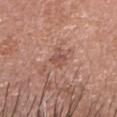The lesion was tiled from a total-body skin photograph and was not biopsied.
Captured under white-light illumination.
A close-up tile cropped from a whole-body skin photograph, about 15 mm across.
The patient is a female in their mid-70s.
Longest diameter approximately 2.5 mm.
The lesion is on the head or neck.